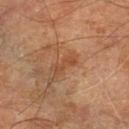- diameter: ~3 mm (longest diameter)
- subject: male, in their 70s
- illumination: cross-polarized illumination
- acquisition: 15 mm crop, total-body photography
- body site: the left lower leg